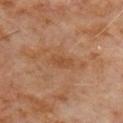workup — catalogued during a skin exam; not biopsied | anatomic site — the front of the torso | image source — total-body-photography crop, ~15 mm field of view | subject — male, aged approximately 60.